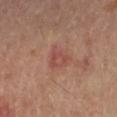• follow-up — imaged on a skin check; not biopsied